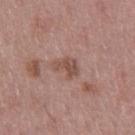biopsy status: imaged on a skin check; not biopsied